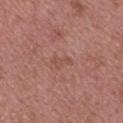Impression:
The lesion was photographed on a routine skin check and not biopsied; there is no pathology result.
Acquisition and patient details:
The subject is a female about 50 years old. Cropped from a whole-body photographic skin survey; the tile spans about 15 mm. The lesion is located on the upper back.